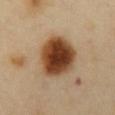biopsy_status: not biopsied; imaged during a skin examination
automated_metrics:
  area_mm2_approx: 20.0
  eccentricity: 0.55
  shape_asymmetry: 0.15
  nevus_likeness_0_100: 100
  lesion_detection_confidence_0_100: 100
lighting: cross-polarized
site: abdomen
image:
  source: total-body photography crop
  field_of_view_mm: 15
lesion_size:
  long_diameter_mm_approx: 5.5
patient:
  sex: female
  age_approx: 60